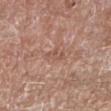follow-up: imaged on a skin check; not biopsied
lighting: white-light illumination
image: total-body-photography crop, ~15 mm field of view
lesion diameter: about 2.5 mm
patient: male, aged around 60
location: the right lower leg
automated lesion analysis: a classifier nevus-likeness of about 0/100 and a detector confidence of about 100 out of 100 that the crop contains a lesion